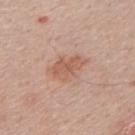Recorded during total-body skin imaging; not selected for excision or biopsy. The recorded lesion diameter is about 4 mm. A 15 mm close-up tile from a total-body photography series done for melanoma screening. The lesion is on the back. The total-body-photography lesion software estimated an eccentricity of roughly 0.8 and two-axis asymmetry of about 0.3. It also reported about 9 CIELAB-L* units darker than the surrounding skin and a lesion-to-skin contrast of about 6.5 (normalized; higher = more distinct). It also reported border irregularity of about 3.5 on a 0–10 scale, internal color variation of about 2 on a 0–10 scale, and a peripheral color-asymmetry measure near 0.5. Imaged with white-light lighting. A male patient, aged around 50.